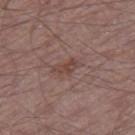Assessment:
The lesion was photographed on a routine skin check and not biopsied; there is no pathology result.
Image and clinical context:
Measured at roughly 3.5 mm in maximum diameter. The lesion is on the right thigh. Cropped from a whole-body photographic skin survey; the tile spans about 15 mm. An algorithmic analysis of the crop reported a footprint of about 4 mm² and two-axis asymmetry of about 0.35. The software also gave a border-irregularity index near 3.5/10, internal color variation of about 2.5 on a 0–10 scale, and peripheral color asymmetry of about 1. Imaged with white-light lighting. A male subject, roughly 65 years of age.